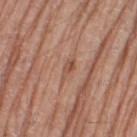The lesion was photographed on a routine skin check and not biopsied; there is no pathology result.
On the right thigh.
Automated image analysis of the tile measured roughly 8 lightness units darker than nearby skin and a lesion-to-skin contrast of about 6 (normalized; higher = more distinct). The software also gave internal color variation of about 1 on a 0–10 scale and peripheral color asymmetry of about 0.
Imaged with white-light lighting.
The lesion's longest dimension is about 2.5 mm.
A 15 mm close-up extracted from a 3D total-body photography capture.
A male subject, roughly 70 years of age.